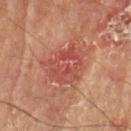Notes:
- follow-up · no biopsy performed (imaged during a skin exam)
- anatomic site · the left upper arm
- size · ≈4.5 mm
- patient · male, aged around 75
- imaging modality · ~15 mm tile from a whole-body skin photo